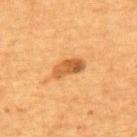Clinical impression:
The lesion was tiled from a total-body skin photograph and was not biopsied.
Acquisition and patient details:
The subject is a female approximately 60 years of age. This is a cross-polarized tile. Longest diameter approximately 4 mm. Cropped from a whole-body photographic skin survey; the tile spans about 15 mm. From the upper back.